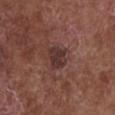Q: What are the patient's age and sex?
A: female, approximately 80 years of age
Q: How was this image acquired?
A: ~15 mm tile from a whole-body skin photo
Q: What is the anatomic site?
A: the chest